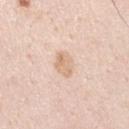| key | value |
|---|---|
| follow-up | total-body-photography surveillance lesion; no biopsy |
| lesion diameter | about 3.5 mm |
| site | the left upper arm |
| subject | male, in their mid- to late 30s |
| illumination | white-light illumination |
| image | total-body-photography crop, ~15 mm field of view |
| TBP lesion metrics | a lesion–skin lightness drop of about 8 and a lesion-to-skin contrast of about 6 (normalized; higher = more distinct) |